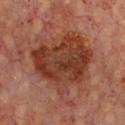follow-up: imaged on a skin check; not biopsied | lighting: cross-polarized illumination | subject: about 65 years old | site: the chest | size: about 7.5 mm | automated lesion analysis: a classifier nevus-likeness of about 20/100 and a detector confidence of about 100 out of 100 that the crop contains a lesion | image source: ~15 mm crop, total-body skin-cancer survey.